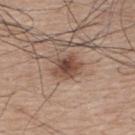biopsy_status: not biopsied; imaged during a skin examination
site: back
automated_metrics:
  cielab_L: 47
  cielab_a: 19
  cielab_b: 26
  vs_skin_darker_L: 12.0
  vs_skin_contrast_norm: 8.5
  border_irregularity_0_10: 2.5
  color_variation_0_10: 5.0
  peripheral_color_asymmetry: 1.5
lesion_size:
  long_diameter_mm_approx: 3.5
image:
  source: total-body photography crop
  field_of_view_mm: 15
patient:
  sex: male
  age_approx: 75